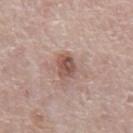  biopsy_status: not biopsied; imaged during a skin examination
  patient:
    sex: male
    age_approx: 70
  image:
    source: total-body photography crop
    field_of_view_mm: 15
  site: chest
  lighting: white-light
  automated_metrics:
    vs_skin_darker_L: 12.0
  lesion_size:
    long_diameter_mm_approx: 3.0